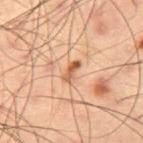Imaged during a routine full-body skin examination; the lesion was not biopsied and no histopathology is available.
A 15 mm close-up extracted from a 3D total-body photography capture.
About 2.5 mm across.
A male patient, aged 53–57.
Located on the leg.
This is a cross-polarized tile.